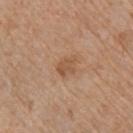Findings:
* follow-up — imaged on a skin check; not biopsied
* location — the right upper arm
* patient — male, aged approximately 70
* image — total-body-photography crop, ~15 mm field of view